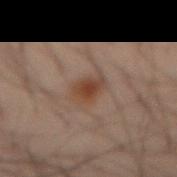workup = no biopsy performed (imaged during a skin exam); subject = male, roughly 40 years of age; acquisition = ~15 mm tile from a whole-body skin photo; lesion diameter = about 2.5 mm; anatomic site = the abdomen.